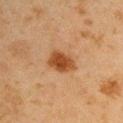Assessment: This lesion was catalogued during total-body skin photography and was not selected for biopsy. Clinical summary: A 15 mm close-up extracted from a 3D total-body photography capture. Approximately 4 mm at its widest. A male patient approximately 45 years of age. Captured under cross-polarized illumination. On the right upper arm.